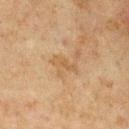follow-up = total-body-photography surveillance lesion; no biopsy | size = about 3 mm | image = ~15 mm tile from a whole-body skin photo | subject = male, aged approximately 75 | lighting = cross-polarized | location = the chest.